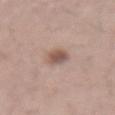Imaged during a routine full-body skin examination; the lesion was not biopsied and no histopathology is available.
The patient is a male in their mid- to late 60s.
Captured under white-light illumination.
A lesion tile, about 15 mm wide, cut from a 3D total-body photograph.
The lesion's longest dimension is about 3 mm.
Automated tile analysis of the lesion measured a lesion color around L≈54 a*≈18 b*≈24 in CIELAB, about 12 CIELAB-L* units darker than the surrounding skin, and a normalized lesion–skin contrast near 8.
From the mid back.